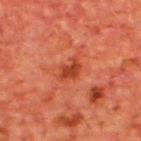workup = total-body-photography surveillance lesion; no biopsy | subject = male, aged 63–67 | site = the upper back | acquisition = ~15 mm crop, total-body skin-cancer survey.